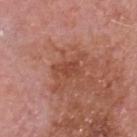Imaged with white-light lighting.
The lesion is on the head or neck.
The recorded lesion diameter is about 3 mm.
An algorithmic analysis of the crop reported a lesion color around L≈46 a*≈27 b*≈31 in CIELAB, about 8 CIELAB-L* units darker than the surrounding skin, and a normalized border contrast of about 6. And it measured a nevus-likeness score of about 0/100 and a lesion-detection confidence of about 100/100.
The patient is a male aged 78 to 82.
Cropped from a total-body skin-imaging series; the visible field is about 15 mm.